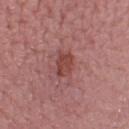Notes:
* biopsy status — catalogued during a skin exam; not biopsied
* location — the right lower leg
* patient — female, aged 48 to 52
* image — ~15 mm crop, total-body skin-cancer survey